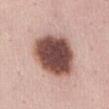Part of a total-body skin-imaging series; this lesion was reviewed on a skin check and was not flagged for biopsy. About 6 mm across. A 15 mm close-up tile from a total-body photography series done for melanoma screening. On the abdomen. The patient is a female approximately 45 years of age. The lesion-visualizer software estimated a lesion area of about 28 mm², an outline eccentricity of about 0.4 (0 = round, 1 = elongated), and a symmetry-axis asymmetry near 0.15.